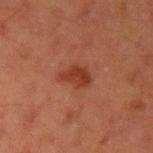biopsy_status: not biopsied; imaged during a skin examination
image:
  source: total-body photography crop
  field_of_view_mm: 15
lighting: cross-polarized
automated_metrics:
  shape_asymmetry: 0.3
  cielab_L: 32
  cielab_a: 25
  cielab_b: 28
  vs_skin_darker_L: 8.0
  vs_skin_contrast_norm: 7.5
  border_irregularity_0_10: 3.0
  color_variation_0_10: 2.5
  peripheral_color_asymmetry: 1.0
lesion_size:
  long_diameter_mm_approx: 3.5
site: left upper arm
patient:
  sex: male
  age_approx: 45